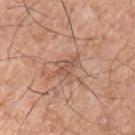{
  "biopsy_status": "not biopsied; imaged during a skin examination",
  "image": {
    "source": "total-body photography crop",
    "field_of_view_mm": 15
  },
  "site": "front of the torso",
  "patient": {
    "sex": "male",
    "age_approx": 75
  },
  "automated_metrics": {
    "area_mm2_approx": 2.5,
    "eccentricity": 0.95,
    "shape_asymmetry": 0.4,
    "cielab_L": 54,
    "cielab_a": 21,
    "cielab_b": 30,
    "vs_skin_darker_L": 8.0,
    "nevus_likeness_0_100": 0,
    "lesion_detection_confidence_0_100": 75
  },
  "lighting": "white-light"
}